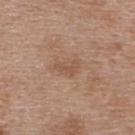Recorded during total-body skin imaging; not selected for excision or biopsy. The patient is a female roughly 40 years of age. The lesion is located on the upper back. The lesion's longest dimension is about 3 mm. A 15 mm close-up extracted from a 3D total-body photography capture. An algorithmic analysis of the crop reported a border-irregularity rating of about 3.5/10 and radial color variation of about 0.5. The tile uses white-light illumination.